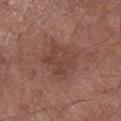Assessment:
Captured during whole-body skin photography for melanoma surveillance; the lesion was not biopsied.
Context:
A male subject in their mid-50s. On the right lower leg. The lesion's longest dimension is about 4 mm. A region of skin cropped from a whole-body photographic capture, roughly 15 mm wide. The total-body-photography lesion software estimated a footprint of about 11 mm², an eccentricity of roughly 0.3, and a shape-asymmetry score of about 0.3 (0 = symmetric). It also reported an average lesion color of about L≈42 a*≈21 b*≈24 (CIELAB), a lesion–skin lightness drop of about 7, and a lesion-to-skin contrast of about 5.5 (normalized; higher = more distinct). The analysis additionally found a nevus-likeness score of about 0/100 and lesion-presence confidence of about 100/100.